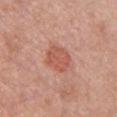The lesion was photographed on a routine skin check and not biopsied; there is no pathology result. Cropped from a total-body skin-imaging series; the visible field is about 15 mm. The lesion is located on the chest. A female patient aged 53–57. Approximately 3.5 mm at its widest.